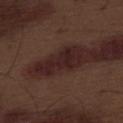This lesion was catalogued during total-body skin photography and was not selected for biopsy.
This image is a 15 mm lesion crop taken from a total-body photograph.
The patient is a male aged 68–72.
On the abdomen.